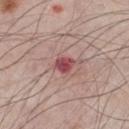Q: Was this lesion biopsied?
A: imaged on a skin check; not biopsied
Q: Who is the patient?
A: male, aged 63 to 67
Q: What is the anatomic site?
A: the chest
Q: What did automated image analysis measure?
A: an area of roughly 4 mm² and a symmetry-axis asymmetry near 0.2; a mean CIELAB color near L≈48 a*≈30 b*≈19 and roughly 13 lightness units darker than nearby skin; a border-irregularity index near 2/10, internal color variation of about 3.5 on a 0–10 scale, and a peripheral color-asymmetry measure near 1; an automated nevus-likeness rating near 0 out of 100 and a lesion-detection confidence of about 100/100
Q: Lesion size?
A: ~2.5 mm (longest diameter)
Q: What lighting was used for the tile?
A: white-light
Q: How was this image acquired?
A: ~15 mm tile from a whole-body skin photo